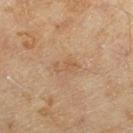This lesion was catalogued during total-body skin photography and was not selected for biopsy. Measured at roughly 3 mm in maximum diameter. Automated tile analysis of the lesion measured a footprint of about 3.5 mm², an eccentricity of roughly 0.85, and a shape-asymmetry score of about 0.4 (0 = symmetric). The analysis additionally found an average lesion color of about L≈51 a*≈17 b*≈31 (CIELAB), roughly 6 lightness units darker than nearby skin, and a normalized lesion–skin contrast near 4.5. The analysis additionally found a classifier nevus-likeness of about 0/100. The subject is a female roughly 60 years of age. A close-up tile cropped from a whole-body skin photograph, about 15 mm across. On the right lower leg.